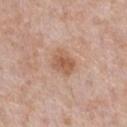automated metrics=an area of roughly 6.5 mm² and an eccentricity of roughly 0.6; patient=male, aged approximately 60; site=the chest; image=total-body-photography crop, ~15 mm field of view; lighting=white-light.